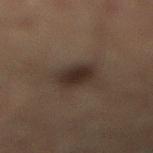Impression: The lesion was photographed on a routine skin check and not biopsied; there is no pathology result. Clinical summary: About 3.5 mm across. A male subject, in their mid- to late 50s. The lesion is located on the left lower leg. A region of skin cropped from a whole-body photographic capture, roughly 15 mm wide. This is a cross-polarized tile.